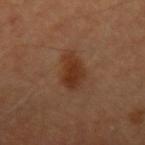Clinical impression:
Recorded during total-body skin imaging; not selected for excision or biopsy.
Background:
The lesion is located on the left upper arm. A male subject, aged approximately 65. A roughly 15 mm field-of-view crop from a total-body skin photograph.